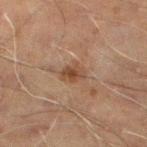This lesion was catalogued during total-body skin photography and was not selected for biopsy.
Longest diameter approximately 3 mm.
On the left lower leg.
The subject is a male about 60 years old.
Cropped from a total-body skin-imaging series; the visible field is about 15 mm.
The tile uses cross-polarized illumination.